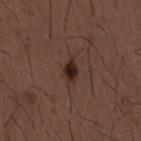Clinical impression:
Imaged during a routine full-body skin examination; the lesion was not biopsied and no histopathology is available.
Clinical summary:
A 15 mm close-up tile from a total-body photography series done for melanoma screening. From the lower back. The subject is a male about 50 years old.